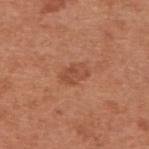Impression: Recorded during total-body skin imaging; not selected for excision or biopsy. Clinical summary: This image is a 15 mm lesion crop taken from a total-body photograph. On the upper back. The total-body-photography lesion software estimated an average lesion color of about L≈49 a*≈26 b*≈33 (CIELAB), about 8 CIELAB-L* units darker than the surrounding skin, and a normalized lesion–skin contrast near 5.5. The recorded lesion diameter is about 3 mm. The subject is a male in their mid-50s. Imaged with white-light lighting.